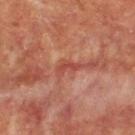Q: Is there a histopathology result?
A: no biopsy performed (imaged during a skin exam)
Q: How large is the lesion?
A: ~3 mm (longest diameter)
Q: What lighting was used for the tile?
A: cross-polarized
Q: What kind of image is this?
A: ~15 mm tile from a whole-body skin photo
Q: Patient demographics?
A: male, in their mid- to late 50s
Q: What is the anatomic site?
A: the upper back